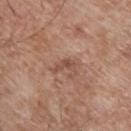<case>
<lighting>white-light</lighting>
<lesion_size>
  <long_diameter_mm_approx>3.0</long_diameter_mm_approx>
</lesion_size>
<patient>
  <sex>male</sex>
  <age_approx>65</age_approx>
</patient>
<site>upper back</site>
<image>
  <source>total-body photography crop</source>
  <field_of_view_mm>15</field_of_view_mm>
</image>
</case>